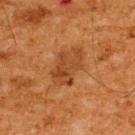Captured during whole-body skin photography for melanoma surveillance; the lesion was not biopsied. The lesion is on the upper back. The subject is a male aged 63 to 67. Cropped from a whole-body photographic skin survey; the tile spans about 15 mm. Captured under cross-polarized illumination. About 5 mm across.